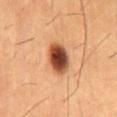{"biopsy_status": "not biopsied; imaged during a skin examination", "lighting": "cross-polarized", "patient": {"sex": "male", "age_approx": 55}, "image": {"source": "total-body photography crop", "field_of_view_mm": 15}, "site": "abdomen"}